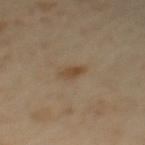Recorded during total-body skin imaging; not selected for excision or biopsy.